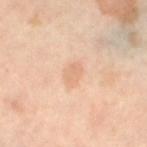biopsy_status: not biopsied; imaged during a skin examination
site: leg
automated_metrics:
  nevus_likeness_0_100: 0
  lesion_detection_confidence_0_100: 100
lesion_size:
  long_diameter_mm_approx: 3.0
patient:
  sex: female
  age_approx: 50
lighting: cross-polarized
image:
  source: total-body photography crop
  field_of_view_mm: 15